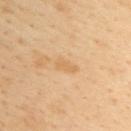Clinical impression: Imaged during a routine full-body skin examination; the lesion was not biopsied and no histopathology is available. Image and clinical context: Cropped from a whole-body photographic skin survey; the tile spans about 15 mm. A male patient, about 40 years old. Located on the upper back.